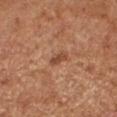biopsy status: total-body-photography surveillance lesion; no biopsy | body site: the right forearm | imaging modality: ~15 mm crop, total-body skin-cancer survey | tile lighting: cross-polarized illumination | image-analysis metrics: a border-irregularity index near 3.5/10, a within-lesion color-variation index near 0.5/10, and peripheral color asymmetry of about 0; a classifier nevus-likeness of about 25/100 and a lesion-detection confidence of about 100/100 | lesion diameter: about 2.5 mm | subject: female, in their 50s.